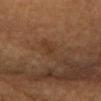Recorded during total-body skin imaging; not selected for excision or biopsy.
This image is a 15 mm lesion crop taken from a total-body photograph.
Located on the head or neck.
The subject is a female approximately 70 years of age.